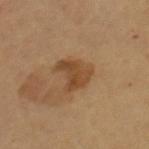notes = no biopsy performed (imaged during a skin exam) | body site = the right upper arm | patient = female, aged 68–72 | acquisition = 15 mm crop, total-body photography.